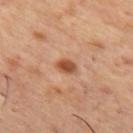Assessment: No biopsy was performed on this lesion — it was imaged during a full skin examination and was not determined to be concerning.